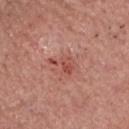Imaged during a routine full-body skin examination; the lesion was not biopsied and no histopathology is available. A male patient, about 80 years old. On the chest. A region of skin cropped from a whole-body photographic capture, roughly 15 mm wide.